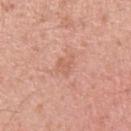Findings:
* notes: imaged on a skin check; not biopsied
* imaging modality: 15 mm crop, total-body photography
* automated metrics: an area of roughly 4 mm²; an automated nevus-likeness rating near 0 out of 100 and lesion-presence confidence of about 100/100
* lighting: white-light
* patient: male, roughly 35 years of age
* location: the right upper arm